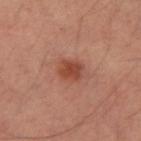{"biopsy_status": "not biopsied; imaged during a skin examination", "image": {"source": "total-body photography crop", "field_of_view_mm": 15}, "lighting": "cross-polarized", "patient": {"sex": "male", "age_approx": 35}, "lesion_size": {"long_diameter_mm_approx": 3.0}, "site": "right upper arm"}